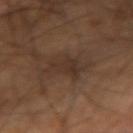biopsy_status: not biopsied; imaged during a skin examination
patient:
  age_approx: 65
lighting: cross-polarized
image:
  source: total-body photography crop
  field_of_view_mm: 15
site: arm